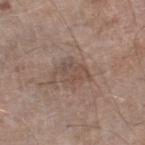Assessment:
The lesion was photographed on a routine skin check and not biopsied; there is no pathology result.
Acquisition and patient details:
The lesion is on the right lower leg. Cropped from a whole-body photographic skin survey; the tile spans about 15 mm. A male subject aged around 60. Imaged with white-light lighting.